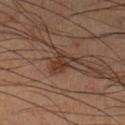Imaged during a routine full-body skin examination; the lesion was not biopsied and no histopathology is available.
The lesion is on the leg.
The lesion-visualizer software estimated border irregularity of about 3.5 on a 0–10 scale and internal color variation of about 3 on a 0–10 scale.
A close-up tile cropped from a whole-body skin photograph, about 15 mm across.
The recorded lesion diameter is about 3 mm.
A male subject roughly 60 years of age.